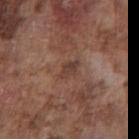A male subject approximately 75 years of age. Automated tile analysis of the lesion measured an average lesion color of about L≈39 a*≈19 b*≈23 (CIELAB), roughly 8 lightness units darker than nearby skin, and a normalized lesion–skin contrast near 6.5. The software also gave border irregularity of about 2.5 on a 0–10 scale, internal color variation of about 2 on a 0–10 scale, and peripheral color asymmetry of about 1. The software also gave an automated nevus-likeness rating near 0 out of 100 and a detector confidence of about 100 out of 100 that the crop contains a lesion. This is a white-light tile. Cropped from a total-body skin-imaging series; the visible field is about 15 mm. The lesion is on the chest.